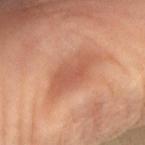Clinical impression:
Captured during whole-body skin photography for melanoma surveillance; the lesion was not biopsied.
Image and clinical context:
A female patient, aged around 50. An algorithmic analysis of the crop reported a symmetry-axis asymmetry near 0.2. The analysis additionally found a lesion color around L≈56 a*≈26 b*≈33 in CIELAB, about 8 CIELAB-L* units darker than the surrounding skin, and a lesion-to-skin contrast of about 5.5 (normalized; higher = more distinct). It also reported a nevus-likeness score of about 0/100 and a lesion-detection confidence of about 100/100. The tile uses cross-polarized illumination. Measured at roughly 6.5 mm in maximum diameter. Cropped from a total-body skin-imaging series; the visible field is about 15 mm. The lesion is on the right forearm.